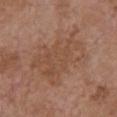<tbp_lesion>
<biopsy_status>not biopsied; imaged during a skin examination</biopsy_status>
<lighting>white-light</lighting>
<image>
  <source>total-body photography crop</source>
  <field_of_view_mm>15</field_of_view_mm>
</image>
<site>chest</site>
<patient>
  <sex>female</sex>
  <age_approx>65</age_approx>
</patient>
<automated_metrics>
  <area_mm2_approx>29.0</area_mm2_approx>
  <eccentricity>0.75</eccentricity>
  <shape_asymmetry>0.3</shape_asymmetry>
  <cielab_L>49</cielab_L>
  <cielab_a>20</cielab_a>
  <cielab_b>29</cielab_b>
  <vs_skin_darker_L>6.0</vs_skin_darker_L>
  <vs_skin_contrast_norm>5.0</vs_skin_contrast_norm>
  <border_irregularity_0_10>5.0</border_irregularity_0_10>
  <color_variation_0_10>3.0</color_variation_0_10>
  <nevus_likeness_0_100>0</nevus_likeness_0_100>
  <lesion_detection_confidence_0_100>100</lesion_detection_confidence_0_100>
</automated_metrics>
</tbp_lesion>